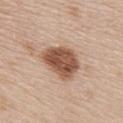Recorded during total-body skin imaging; not selected for excision or biopsy. Automated tile analysis of the lesion measured an area of roughly 14 mm² and a shape-asymmetry score of about 0.25 (0 = symmetric). The analysis additionally found border irregularity of about 2.5 on a 0–10 scale and radial color variation of about 1.5. The software also gave an automated nevus-likeness rating near 80 out of 100 and a detector confidence of about 100 out of 100 that the crop contains a lesion. The lesion is located on the upper back. The subject is a female aged 48–52. The recorded lesion diameter is about 4.5 mm. A close-up tile cropped from a whole-body skin photograph, about 15 mm across. This is a white-light tile.